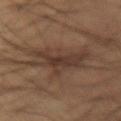| feature | finding |
|---|---|
| notes | total-body-photography surveillance lesion; no biopsy |
| illumination | cross-polarized |
| site | the right forearm |
| diameter | about 5.5 mm |
| imaging modality | 15 mm crop, total-body photography |
| patient | male, aged approximately 60 |
| image-analysis metrics | a lesion area of about 17 mm², an eccentricity of roughly 0.6, and a symmetry-axis asymmetry near 0.4; border irregularity of about 8 on a 0–10 scale and radial color variation of about 0.5 |